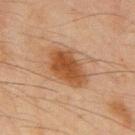notes: catalogued during a skin exam; not biopsied | lesion size: about 5 mm | body site: the back | patient: male, in their mid- to late 40s | image: 15 mm crop, total-body photography.